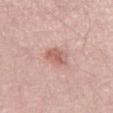Findings:
* lesion size — ≈3 mm
* image source — 15 mm crop, total-body photography
* automated lesion analysis — a lesion area of about 5 mm²; an automated nevus-likeness rating near 60 out of 100
* anatomic site — the left thigh
* subject — male, aged 48–52
* tile lighting — white-light